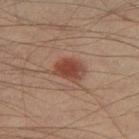* image source — ~15 mm tile from a whole-body skin photo
* patient — male, aged 38 to 42
* lighting — cross-polarized illumination
* body site — the left thigh